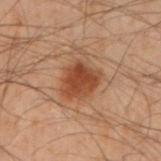Imaged with cross-polarized lighting.
The total-body-photography lesion software estimated a lesion color around L≈37 a*≈21 b*≈29 in CIELAB and a lesion–skin lightness drop of about 10. And it measured a within-lesion color-variation index near 3.5/10 and a peripheral color-asymmetry measure near 1.
Located on the left forearm.
A male patient, aged approximately 50.
A roughly 15 mm field-of-view crop from a total-body skin photograph.
The recorded lesion diameter is about 5 mm.